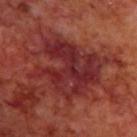Q: Was a biopsy performed?
A: total-body-photography surveillance lesion; no biopsy
Q: Lesion location?
A: the back
Q: Who is the patient?
A: male, aged 68–72
Q: Automated lesion metrics?
A: a lesion area of about 33 mm² and a shape eccentricity near 0.6
Q: What is the imaging modality?
A: 15 mm crop, total-body photography